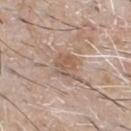Q: What kind of image is this?
A: 15 mm crop, total-body photography
Q: Lesion location?
A: the chest
Q: Who is the patient?
A: male, about 65 years old